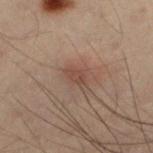Q: Was this lesion biopsied?
A: catalogued during a skin exam; not biopsied
Q: What is the anatomic site?
A: the leg
Q: How was the tile lit?
A: cross-polarized
Q: How was this image acquired?
A: ~15 mm tile from a whole-body skin photo
Q: Patient demographics?
A: male, about 55 years old
Q: How large is the lesion?
A: ≈3 mm